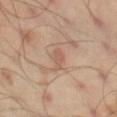workup — catalogued during a skin exam; not biopsied
diameter — about 2.5 mm
image — ~15 mm tile from a whole-body skin photo
tile lighting — cross-polarized illumination
site — the left thigh
patient — male, aged around 45